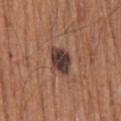This lesion was catalogued during total-body skin photography and was not selected for biopsy.
The lesion's longest dimension is about 3.5 mm.
An algorithmic analysis of the crop reported a border-irregularity index near 2.5/10, a within-lesion color-variation index near 5.5/10, and peripheral color asymmetry of about 1.5.
Imaged with white-light lighting.
Located on the mid back.
A male subject in their mid- to late 60s.
Cropped from a total-body skin-imaging series; the visible field is about 15 mm.